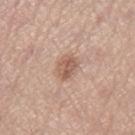Case summary:
• follow-up: imaged on a skin check; not biopsied
• location: the left thigh
• automated lesion analysis: a lesion color around L≈58 a*≈19 b*≈28 in CIELAB and a normalized border contrast of about 7; a classifier nevus-likeness of about 55/100 and lesion-presence confidence of about 100/100
• patient: female, aged 63–67
• lesion size: about 3 mm
• image: 15 mm crop, total-body photography